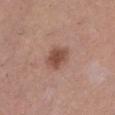follow-up — total-body-photography surveillance lesion; no biopsy
illumination — white-light illumination
patient — female, aged 28 to 32
image source — 15 mm crop, total-body photography
diameter — ~3 mm (longest diameter)
body site — the leg